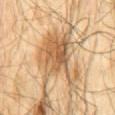Q: Was this lesion biopsied?
A: no biopsy performed (imaged during a skin exam)
Q: What are the patient's age and sex?
A: male, approximately 65 years of age
Q: What is the imaging modality?
A: ~15 mm tile from a whole-body skin photo
Q: How was the tile lit?
A: cross-polarized illumination
Q: Where on the body is the lesion?
A: the mid back
Q: How large is the lesion?
A: ~8.5 mm (longest diameter)
Q: What did automated image analysis measure?
A: an area of roughly 25 mm², a shape eccentricity near 0.8, and two-axis asymmetry of about 0.45; a mean CIELAB color near L≈57 a*≈17 b*≈36, a lesion–skin lightness drop of about 12, and a lesion-to-skin contrast of about 8 (normalized; higher = more distinct); a color-variation rating of about 6.5/10 and a peripheral color-asymmetry measure near 2; a classifier nevus-likeness of about 20/100 and a detector confidence of about 95 out of 100 that the crop contains a lesion